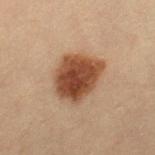This lesion was catalogued during total-body skin photography and was not selected for biopsy. Located on the right thigh. A 15 mm crop from a total-body photograph taken for skin-cancer surveillance. The total-body-photography lesion software estimated a footprint of about 17 mm². A female subject about 30 years old. This is a cross-polarized tile.